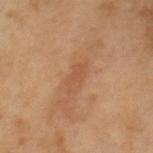  biopsy_status: not biopsied; imaged during a skin examination
  lesion_size:
    long_diameter_mm_approx: 3.5
  image:
    source: total-body photography crop
    field_of_view_mm: 15
  automated_metrics:
    border_irregularity_0_10: 5.5
    peripheral_color_asymmetry: 0.0
    nevus_likeness_0_100: 0
    lesion_detection_confidence_0_100: 100
  lighting: cross-polarized
  patient:
    sex: male
    age_approx: 65